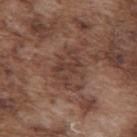Assessment: Recorded during total-body skin imaging; not selected for excision or biopsy. Acquisition and patient details: Imaged with white-light lighting. A male subject aged approximately 75. The recorded lesion diameter is about 5 mm. Cropped from a total-body skin-imaging series; the visible field is about 15 mm. The lesion is located on the mid back.